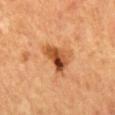Clinical impression:
Part of a total-body skin-imaging series; this lesion was reviewed on a skin check and was not flagged for biopsy.